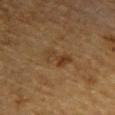follow-up: total-body-photography surveillance lesion; no biopsy | diameter: about 4 mm | body site: the right upper arm | patient: male, aged approximately 85 or older | automated metrics: an area of roughly 6.5 mm², an eccentricity of roughly 0.85, and a symmetry-axis asymmetry near 0.3; a lesion color around L≈33 a*≈15 b*≈29 in CIELAB and a lesion-to-skin contrast of about 6 (normalized; higher = more distinct); a nevus-likeness score of about 5/100 and a detector confidence of about 100 out of 100 that the crop contains a lesion | illumination: cross-polarized | acquisition: ~15 mm crop, total-body skin-cancer survey.